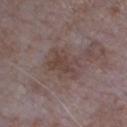Recorded during total-body skin imaging; not selected for excision or biopsy. The patient is a male in their mid-60s. The lesion's longest dimension is about 4 mm. Automated image analysis of the tile measured a shape eccentricity near 0.65. And it measured an average lesion color of about L≈42 a*≈15 b*≈20 (CIELAB), a lesion–skin lightness drop of about 6, and a lesion-to-skin contrast of about 6 (normalized; higher = more distinct). And it measured border irregularity of about 5.5 on a 0–10 scale, a color-variation rating of about 3/10, and radial color variation of about 1. A close-up tile cropped from a whole-body skin photograph, about 15 mm across. On the chest.